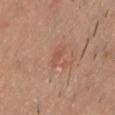workup = catalogued during a skin exam; not biopsied
image = total-body-photography crop, ~15 mm field of view
lesion diameter = about 2.5 mm
subject = male, aged approximately 35
body site = the front of the torso